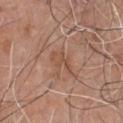No biopsy was performed on this lesion — it was imaged during a full skin examination and was not determined to be concerning.
The lesion-visualizer software estimated a footprint of about 4 mm². It also reported a lesion color around L≈51 a*≈20 b*≈30 in CIELAB, a lesion–skin lightness drop of about 6, and a normalized lesion–skin contrast near 5.5. The analysis additionally found a border-irregularity rating of about 6/10 and a color-variation rating of about 0.5/10. And it measured a nevus-likeness score of about 0/100.
Imaged with white-light lighting.
The lesion is on the front of the torso.
Longest diameter approximately 3.5 mm.
The subject is a male roughly 80 years of age.
This image is a 15 mm lesion crop taken from a total-body photograph.